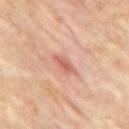Clinical impression: Part of a total-body skin-imaging series; this lesion was reviewed on a skin check and was not flagged for biopsy. Acquisition and patient details: Approximately 2.5 mm at its widest. Captured under cross-polarized illumination. Automated tile analysis of the lesion measured a border-irregularity rating of about 4/10 and radial color variation of about 0. The analysis additionally found an automated nevus-likeness rating near 0 out of 100. A 15 mm close-up extracted from a 3D total-body photography capture. The lesion is on the mid back. The subject is a male aged around 60.